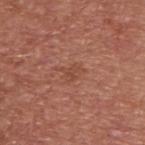Assessment:
Imaged during a routine full-body skin examination; the lesion was not biopsied and no histopathology is available.
Background:
The lesion is on the back. Imaged with white-light lighting. This image is a 15 mm lesion crop taken from a total-body photograph. A male subject, aged approximately 65. Automated tile analysis of the lesion measured a lesion area of about 4 mm², an eccentricity of roughly 0.65, and a shape-asymmetry score of about 0.5 (0 = symmetric). The analysis additionally found a mean CIELAB color near L≈47 a*≈25 b*≈30, roughly 6 lightness units darker than nearby skin, and a normalized lesion–skin contrast near 5. The software also gave a classifier nevus-likeness of about 0/100.